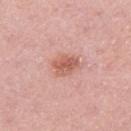Impression: No biopsy was performed on this lesion — it was imaged during a full skin examination and was not determined to be concerning. Clinical summary: Cropped from a total-body skin-imaging series; the visible field is about 15 mm. A female subject, about 30 years old. The recorded lesion diameter is about 3 mm. An algorithmic analysis of the crop reported an average lesion color of about L≈59 a*≈26 b*≈28 (CIELAB) and about 11 CIELAB-L* units darker than the surrounding skin. This is a white-light tile. Located on the arm.